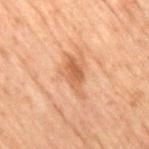Q: Was this lesion biopsied?
A: no biopsy performed (imaged during a skin exam)
Q: How was this image acquired?
A: total-body-photography crop, ~15 mm field of view
Q: What did automated image analysis measure?
A: a detector confidence of about 100 out of 100 that the crop contains a lesion
Q: Where on the body is the lesion?
A: the right thigh
Q: What is the lesion's diameter?
A: about 5 mm
Q: Patient demographics?
A: male, roughly 70 years of age